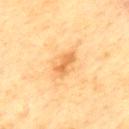Clinical impression: No biopsy was performed on this lesion — it was imaged during a full skin examination and was not determined to be concerning. Context: A 15 mm close-up tile from a total-body photography series done for melanoma screening. Approximately 3.5 mm at its widest. The subject is a male aged approximately 70. This is a cross-polarized tile. From the upper back.